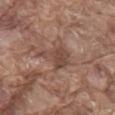Impression:
No biopsy was performed on this lesion — it was imaged during a full skin examination and was not determined to be concerning.
Context:
This image is a 15 mm lesion crop taken from a total-body photograph. A male subject in their 80s. The lesion is located on the mid back.